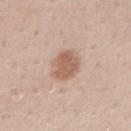Q: Was a biopsy performed?
A: catalogued during a skin exam; not biopsied
Q: How was the tile lit?
A: white-light illumination
Q: What is the lesion's diameter?
A: ≈4 mm
Q: Where on the body is the lesion?
A: the right upper arm
Q: How was this image acquired?
A: 15 mm crop, total-body photography
Q: Patient demographics?
A: male, aged around 60